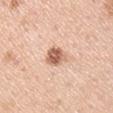This lesion was catalogued during total-body skin photography and was not selected for biopsy.
A roughly 15 mm field-of-view crop from a total-body skin photograph.
A male patient approximately 45 years of age.
Imaged with white-light lighting.
An algorithmic analysis of the crop reported a lesion area of about 6 mm², an outline eccentricity of about 0.65 (0 = round, 1 = elongated), and a shape-asymmetry score of about 0.2 (0 = symmetric). The software also gave an average lesion color of about L≈62 a*≈22 b*≈31 (CIELAB) and a normalized border contrast of about 9.5. It also reported border irregularity of about 2 on a 0–10 scale, internal color variation of about 4.5 on a 0–10 scale, and a peripheral color-asymmetry measure near 1.5.
From the left upper arm.
About 3 mm across.